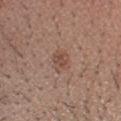Imaged during a routine full-body skin examination; the lesion was not biopsied and no histopathology is available. A male patient in their 30s. Imaged with white-light lighting. A 15 mm close-up extracted from a 3D total-body photography capture. Measured at roughly 3 mm in maximum diameter. Located on the head or neck. An algorithmic analysis of the crop reported internal color variation of about 3 on a 0–10 scale and radial color variation of about 1.